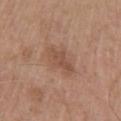Findings:
* biopsy status: no biopsy performed (imaged during a skin exam)
* lighting: white-light
* lesion size: ~4 mm (longest diameter)
* subject: male, roughly 60 years of age
* site: the chest
* image source: ~15 mm crop, total-body skin-cancer survey